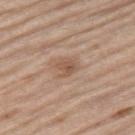Q: Was a biopsy performed?
A: imaged on a skin check; not biopsied
Q: Illumination type?
A: white-light
Q: What kind of image is this?
A: ~15 mm tile from a whole-body skin photo
Q: What are the patient's age and sex?
A: male, roughly 70 years of age
Q: How large is the lesion?
A: ~2.5 mm (longest diameter)
Q: What is the anatomic site?
A: the right thigh